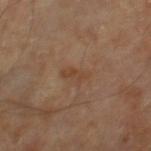Captured during whole-body skin photography for melanoma surveillance; the lesion was not biopsied.
A roughly 15 mm field-of-view crop from a total-body skin photograph.
On the right leg.
A male subject aged 58–62.
The tile uses cross-polarized illumination.
About 3 mm across.